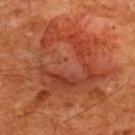No biopsy was performed on this lesion — it was imaged during a full skin examination and was not determined to be concerning. The tile uses cross-polarized illumination. The lesion is located on the upper back. Longest diameter approximately 11.5 mm. A male patient aged around 60. A 15 mm crop from a total-body photograph taken for skin-cancer surveillance.